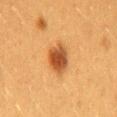Q: What are the patient's age and sex?
A: female, in their 40s
Q: Lesion size?
A: ≈4.5 mm
Q: How was this image acquired?
A: 15 mm crop, total-body photography
Q: What is the anatomic site?
A: the lower back